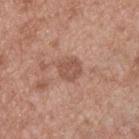Q: How large is the lesion?
A: ≈2.5 mm
Q: What are the patient's age and sex?
A: male, aged approximately 55
Q: What kind of image is this?
A: total-body-photography crop, ~15 mm field of view
Q: What did automated image analysis measure?
A: an area of roughly 5.5 mm², a shape eccentricity near 0.35, and two-axis asymmetry of about 0.2; an average lesion color of about L≈52 a*≈21 b*≈29 (CIELAB) and a normalized lesion–skin contrast near 6.5
Q: Lesion location?
A: the upper back
Q: Illumination type?
A: white-light